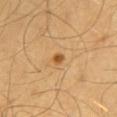This lesion was catalogued during total-body skin photography and was not selected for biopsy. On the mid back. A male patient, aged 43 to 47. A roughly 15 mm field-of-view crop from a total-body skin photograph. The tile uses cross-polarized illumination. Automated image analysis of the tile measured an area of roughly 2 mm², an outline eccentricity of about 0.5 (0 = round, 1 = elongated), and a shape-asymmetry score of about 0.25 (0 = symmetric). The analysis additionally found roughly 12 lightness units darker than nearby skin and a normalized lesion–skin contrast near 9. The software also gave border irregularity of about 2 on a 0–10 scale, a color-variation rating of about 2/10, and a peripheral color-asymmetry measure near 0.5. The software also gave an automated nevus-likeness rating near 90 out of 100. The recorded lesion diameter is about 1.5 mm.